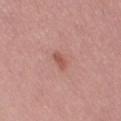notes: no biopsy performed (imaged during a skin exam) | acquisition: ~15 mm crop, total-body skin-cancer survey | size: ~2.5 mm (longest diameter) | patient: female, aged around 70 | lighting: white-light illumination | automated lesion analysis: a footprint of about 3 mm², an outline eccentricity of about 0.85 (0 = round, 1 = elongated), and a shape-asymmetry score of about 0.3 (0 = symmetric); a lesion color around L≈54 a*≈26 b*≈27 in CIELAB, a lesion–skin lightness drop of about 9, and a normalized lesion–skin contrast near 6.5; internal color variation of about 1.5 on a 0–10 scale and radial color variation of about 0.5; a classifier nevus-likeness of about 80/100 and a lesion-detection confidence of about 100/100 | location: the left thigh.